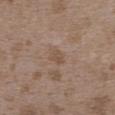No biopsy was performed on this lesion — it was imaged during a full skin examination and was not determined to be concerning. A female patient, approximately 35 years of age. The total-body-photography lesion software estimated a normalized lesion–skin contrast near 5.5. The software also gave a border-irregularity index near 3.5/10 and internal color variation of about 1 on a 0–10 scale. The tile uses white-light illumination. Approximately 2.5 mm at its widest. A 15 mm close-up extracted from a 3D total-body photography capture. Located on the upper back.